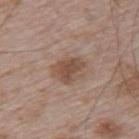Case summary:
- biopsy status — total-body-photography surveillance lesion; no biopsy
- body site — the upper back
- lighting — white-light
- lesion size — about 4 mm
- imaging modality — ~15 mm tile from a whole-body skin photo
- subject — male, about 65 years old